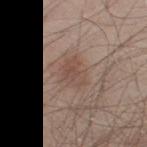Captured during whole-body skin photography for melanoma surveillance; the lesion was not biopsied. Imaged with white-light lighting. Measured at roughly 3.5 mm in maximum diameter. Automated image analysis of the tile measured a border-irregularity rating of about 3/10, a within-lesion color-variation index near 3/10, and peripheral color asymmetry of about 1. A close-up tile cropped from a whole-body skin photograph, about 15 mm across. The lesion is on the right lower leg. A male subject about 35 years old.